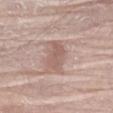Assessment: The lesion was photographed on a routine skin check and not biopsied; there is no pathology result. Clinical summary: The lesion is on the left upper arm. A roughly 15 mm field-of-view crop from a total-body skin photograph. The subject is a male aged around 80.